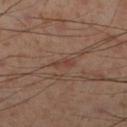Clinical impression:
Captured during whole-body skin photography for melanoma surveillance; the lesion was not biopsied.
Background:
Measured at roughly 2.5 mm in maximum diameter. The lesion is on the right lower leg. An algorithmic analysis of the crop reported a lesion color around L≈41 a*≈20 b*≈25 in CIELAB, roughly 6 lightness units darker than nearby skin, and a normalized border contrast of about 5.5. And it measured a border-irregularity rating of about 4/10, internal color variation of about 0 on a 0–10 scale, and peripheral color asymmetry of about 0. The subject is a male about 55 years old. This is a cross-polarized tile. A 15 mm close-up tile from a total-body photography series done for melanoma screening.